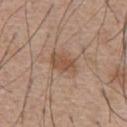Part of a total-body skin-imaging series; this lesion was reviewed on a skin check and was not flagged for biopsy. Cropped from a whole-body photographic skin survey; the tile spans about 15 mm. This is a white-light tile. On the chest. The lesion's longest dimension is about 3.5 mm. The patient is a male approximately 55 years of age. Automated image analysis of the tile measured an area of roughly 6.5 mm², a shape eccentricity near 0.8, and a shape-asymmetry score of about 0.25 (0 = symmetric). And it measured a classifier nevus-likeness of about 50/100.